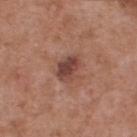Image and clinical context:
On the upper back. About 3 mm across. A 15 mm close-up tile from a total-body photography series done for melanoma screening. Captured under white-light illumination. A male patient, aged 53 to 57.